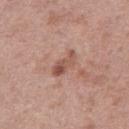<tbp_lesion>
  <biopsy_status>not biopsied; imaged during a skin examination</biopsy_status>
  <site>leg</site>
  <image>
    <source>total-body photography crop</source>
    <field_of_view_mm>15</field_of_view_mm>
  </image>
  <automated_metrics>
    <eccentricity>0.9</eccentricity>
    <shape_asymmetry>0.4</shape_asymmetry>
    <cielab_L>52</cielab_L>
    <cielab_a>22</cielab_a>
    <cielab_b>27</cielab_b>
    <vs_skin_darker_L>11.0</vs_skin_darker_L>
    <vs_skin_contrast_norm>7.5</vs_skin_contrast_norm>
    <border_irregularity_0_10>4.0</border_irregularity_0_10>
    <color_variation_0_10>5.0</color_variation_0_10>
    <peripheral_color_asymmetry>1.5</peripheral_color_asymmetry>
  </automated_metrics>
  <patient>
    <sex>female</sex>
    <age_approx>40</age_approx>
  </patient>
</tbp_lesion>